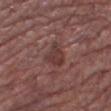• notes — imaged on a skin check; not biopsied
• tile lighting — white-light
• TBP lesion metrics — a nevus-likeness score of about 0/100
• patient — male, aged 63 to 67
• anatomic site — the leg
• image — 15 mm crop, total-body photography